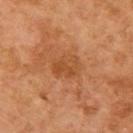Q: Was this lesion biopsied?
A: total-body-photography surveillance lesion; no biopsy
Q: Lesion location?
A: the right upper arm
Q: Patient demographics?
A: female, aged 58 to 62
Q: How was this image acquired?
A: total-body-photography crop, ~15 mm field of view
Q: Lesion size?
A: about 3.5 mm
Q: What lighting was used for the tile?
A: cross-polarized illumination